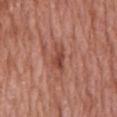Assessment: This lesion was catalogued during total-body skin photography and was not selected for biopsy. Clinical summary: This image is a 15 mm lesion crop taken from a total-body photograph. The lesion's longest dimension is about 4 mm. The lesion is located on the front of the torso. A male subject in their mid-60s. Captured under white-light illumination.